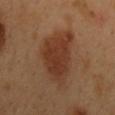follow-up: catalogued during a skin exam; not biopsied | location: the back | imaging modality: 15 mm crop, total-body photography | subject: male, aged 48 to 52 | diameter: ≈8.5 mm | tile lighting: cross-polarized illumination | image-analysis metrics: a footprint of about 24 mm² and a shape-asymmetry score of about 0.3 (0 = symmetric); a lesion color around L≈36 a*≈21 b*≈30 in CIELAB, roughly 9 lightness units darker than nearby skin, and a normalized lesion–skin contrast near 8.5.